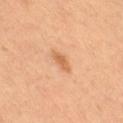Recorded during total-body skin imaging; not selected for excision or biopsy.
This is a cross-polarized tile.
Cropped from a total-body skin-imaging series; the visible field is about 15 mm.
A female subject roughly 55 years of age.
The lesion is located on the right thigh.
The recorded lesion diameter is about 3 mm.